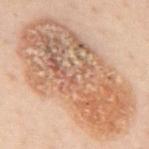| field | value |
|---|---|
| biopsy status | no biopsy performed (imaged during a skin exam) |
| lesion size | about 14.5 mm |
| subject | male, in their 50s |
| image-analysis metrics | a border-irregularity index near 2.5/10, a color-variation rating of about 8/10, and radial color variation of about 2.5; an automated nevus-likeness rating near 65 out of 100 and lesion-presence confidence of about 100/100 |
| anatomic site | the mid back |
| image source | total-body-photography crop, ~15 mm field of view |
| lighting | cross-polarized |